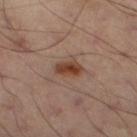| feature | finding |
|---|---|
| follow-up | catalogued during a skin exam; not biopsied |
| anatomic site | the leg |
| TBP lesion metrics | a footprint of about 5 mm² and a shape-asymmetry score of about 0.15 (0 = symmetric); an average lesion color of about L≈41 a*≈20 b*≈28 (CIELAB) and about 11 CIELAB-L* units darker than the surrounding skin; a lesion-detection confidence of about 100/100 |
| illumination | cross-polarized illumination |
| imaging modality | ~15 mm crop, total-body skin-cancer survey |
| size | ~3 mm (longest diameter) |
| subject | male, aged 48 to 52 |